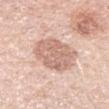notes: catalogued during a skin exam; not biopsied
lighting: white-light
location: the arm
image: ~15 mm crop, total-body skin-cancer survey
subject: female, aged 28–32
image-analysis metrics: an area of roughly 20 mm² and a shape-asymmetry score of about 0.15 (0 = symmetric); border irregularity of about 1.5 on a 0–10 scale, internal color variation of about 3 on a 0–10 scale, and radial color variation of about 1; a classifier nevus-likeness of about 0/100 and lesion-presence confidence of about 100/100